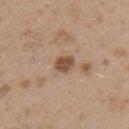body site: the right upper arm | tile lighting: white-light illumination | subject: male, aged around 50 | lesion diameter: ≈2.5 mm | automated lesion analysis: a footprint of about 4.5 mm², an outline eccentricity of about 0.65 (0 = round, 1 = elongated), and a symmetry-axis asymmetry near 0.2; a normalized border contrast of about 9; border irregularity of about 2 on a 0–10 scale, a within-lesion color-variation index near 2.5/10, and radial color variation of about 1 | image: ~15 mm crop, total-body skin-cancer survey.